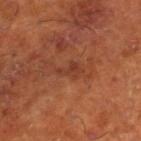follow-up: total-body-photography surveillance lesion; no biopsy | automated metrics: a lesion area of about 3.5 mm² and two-axis asymmetry of about 0.5; internal color variation of about 2 on a 0–10 scale and peripheral color asymmetry of about 1; a nevus-likeness score of about 0/100 and a lesion-detection confidence of about 100/100 | anatomic site: the leg | lesion diameter: ~3.5 mm (longest diameter) | patient: male, roughly 65 years of age | imaging modality: 15 mm crop, total-body photography | illumination: cross-polarized.